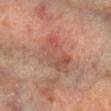imaging modality: ~15 mm crop, total-body skin-cancer survey | lighting: cross-polarized illumination | diameter: ≈5.5 mm | anatomic site: the left forearm | automated metrics: internal color variation of about 7 on a 0–10 scale and radial color variation of about 2.5; a nevus-likeness score of about 0/100 and a lesion-detection confidence of about 100/100 | patient: male, aged approximately 65.